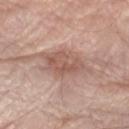Impression:
No biopsy was performed on this lesion — it was imaged during a full skin examination and was not determined to be concerning.
Background:
A male subject, roughly 80 years of age. Longest diameter approximately 6.5 mm. An algorithmic analysis of the crop reported a border-irregularity index near 4.5/10, a within-lesion color-variation index near 3.5/10, and peripheral color asymmetry of about 1.5. The analysis additionally found a nevus-likeness score of about 25/100. A close-up tile cropped from a whole-body skin photograph, about 15 mm across. Captured under white-light illumination. The lesion is on the left forearm.